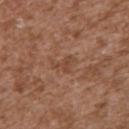The lesion was tiled from a total-body skin photograph and was not biopsied. A male patient, in their mid- to late 40s. A 15 mm crop from a total-body photograph taken for skin-cancer surveillance. The lesion is located on the left upper arm.